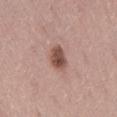The lesion was tiled from a total-body skin photograph and was not biopsied. The lesion's longest dimension is about 3 mm. On the back. The subject is a male approximately 45 years of age. The tile uses white-light illumination. A lesion tile, about 15 mm wide, cut from a 3D total-body photograph.